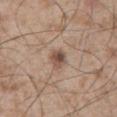Impression: The lesion was photographed on a routine skin check and not biopsied; there is no pathology result. Clinical summary: Located on the abdomen. Automated tile analysis of the lesion measured a lesion area of about 4.5 mm², an eccentricity of roughly 0.65, and a symmetry-axis asymmetry near 0.2. It also reported a normalized lesion–skin contrast near 8. This is a white-light tile. A male subject aged 53 to 57. A region of skin cropped from a whole-body photographic capture, roughly 15 mm wide.